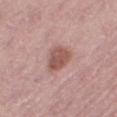{"biopsy_status": "not biopsied; imaged during a skin examination", "patient": {"sex": "female", "age_approx": 50}, "lighting": "white-light", "image": {"source": "total-body photography crop", "field_of_view_mm": 15}, "site": "right thigh", "automated_metrics": {"vs_skin_darker_L": 12.0, "nevus_likeness_0_100": 50, "lesion_detection_confidence_0_100": 100}}